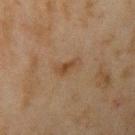- biopsy status — no biopsy performed (imaged during a skin exam)
- image — ~15 mm tile from a whole-body skin photo
- body site — the arm
- lighting — cross-polarized illumination
- lesion diameter — about 3.5 mm
- TBP lesion metrics — a footprint of about 4.5 mm², an eccentricity of roughly 0.9, and two-axis asymmetry of about 0.35; about 6 CIELAB-L* units darker than the surrounding skin and a normalized border contrast of about 6; a border-irregularity rating of about 4/10, internal color variation of about 2.5 on a 0–10 scale, and a peripheral color-asymmetry measure near 0.5; a classifier nevus-likeness of about 5/100 and lesion-presence confidence of about 100/100
- subject — male, roughly 45 years of age